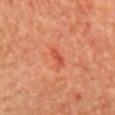The lesion was photographed on a routine skin check and not biopsied; there is no pathology result.
Measured at roughly 2.5 mm in maximum diameter.
A female patient, approximately 55 years of age.
Located on the chest.
This image is a 15 mm lesion crop taken from a total-body photograph.